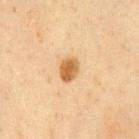Q: Is there a histopathology result?
A: imaged on a skin check; not biopsied
Q: Patient demographics?
A: male, roughly 60 years of age
Q: What lighting was used for the tile?
A: cross-polarized illumination
Q: How large is the lesion?
A: ~2.5 mm (longest diameter)
Q: What is the anatomic site?
A: the chest
Q: What is the imaging modality?
A: total-body-photography crop, ~15 mm field of view
Q: What did automated image analysis measure?
A: a mean CIELAB color near L≈52 a*≈17 b*≈37 and a lesion–skin lightness drop of about 12